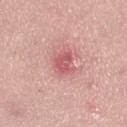The lesion was photographed on a routine skin check and not biopsied; there is no pathology result.
Imaged with white-light lighting.
The lesion is on the right thigh.
The total-body-photography lesion software estimated a normalized lesion–skin contrast near 7. It also reported a border-irregularity rating of about 4/10, internal color variation of about 2 on a 0–10 scale, and peripheral color asymmetry of about 0.5.
Approximately 3 mm at its widest.
A female patient in their mid- to late 20s.
Cropped from a total-body skin-imaging series; the visible field is about 15 mm.